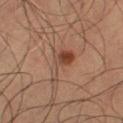Case summary:
– follow-up — total-body-photography surveillance lesion; no biopsy
– automated metrics — a footprint of about 7.5 mm², an outline eccentricity of about 0.85 (0 = round, 1 = elongated), and a symmetry-axis asymmetry near 0.55; a lesion–skin lightness drop of about 9 and a normalized border contrast of about 7; a color-variation rating of about 6/10 and a peripheral color-asymmetry measure near 2
– diameter — about 5 mm
– patient — male, in their mid- to late 60s
– imaging modality — ~15 mm tile from a whole-body skin photo
– illumination — cross-polarized
– body site — the left thigh